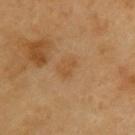Clinical impression:
The lesion was tiled from a total-body skin photograph and was not biopsied.
Context:
Cropped from a total-body skin-imaging series; the visible field is about 15 mm. This is a cross-polarized tile. On the upper back. Automated image analysis of the tile measured a normalized border contrast of about 5. The software also gave a nevus-likeness score of about 0/100 and a detector confidence of about 100 out of 100 that the crop contains a lesion. The patient is a male aged approximately 60.